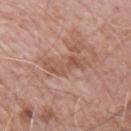Assessment: Part of a total-body skin-imaging series; this lesion was reviewed on a skin check and was not flagged for biopsy. Context: A male patient, in their mid-70s. Cropped from a total-body skin-imaging series; the visible field is about 15 mm. The lesion's longest dimension is about 4.5 mm. Located on the left upper arm. An algorithmic analysis of the crop reported a lesion color around L≈54 a*≈22 b*≈28 in CIELAB, about 7 CIELAB-L* units darker than the surrounding skin, and a normalized lesion–skin contrast near 5.5. And it measured a border-irregularity index near 6.5/10 and radial color variation of about 1. Imaged with white-light lighting.